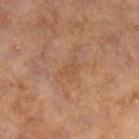Assessment:
Imaged during a routine full-body skin examination; the lesion was not biopsied and no histopathology is available.
Background:
Cropped from a total-body skin-imaging series; the visible field is about 15 mm. A female subject roughly 60 years of age. From the right lower leg. Automated image analysis of the tile measured a lesion color around L≈44 a*≈19 b*≈30 in CIELAB and a normalized lesion–skin contrast near 4.5. The software also gave a classifier nevus-likeness of about 0/100. Approximately 2.5 mm at its widest. The tile uses cross-polarized illumination.